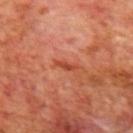The lesion was tiled from a total-body skin photograph and was not biopsied. The recorded lesion diameter is about 2.5 mm. Located on the mid back. Automated image analysis of the tile measured a lesion area of about 2 mm², an eccentricity of roughly 0.95, and a symmetry-axis asymmetry near 0.35. It also reported a classifier nevus-likeness of about 0/100 and a lesion-detection confidence of about 100/100. A region of skin cropped from a whole-body photographic capture, roughly 15 mm wide. The subject is a male approximately 70 years of age. This is a cross-polarized tile.